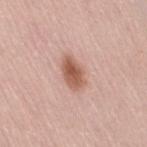The lesion was tiled from a total-body skin photograph and was not biopsied. A region of skin cropped from a whole-body photographic capture, roughly 15 mm wide. Captured under white-light illumination. About 4 mm across. The lesion is on the right thigh. A female patient in their mid-60s.